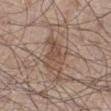The lesion was photographed on a routine skin check and not biopsied; there is no pathology result. A male subject, aged around 55. The lesion is on the right lower leg. A 15 mm crop from a total-body photograph taken for skin-cancer surveillance.